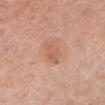follow-up = catalogued during a skin exam; not biopsied
subject = female, aged 63 to 67
size = about 3 mm
site = the head or neck
imaging modality = total-body-photography crop, ~15 mm field of view